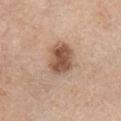This lesion was catalogued during total-body skin photography and was not selected for biopsy.
This is a white-light tile.
The subject is a male aged 58 to 62.
On the chest.
A 15 mm close-up extracted from a 3D total-body photography capture.
About 5.5 mm across.